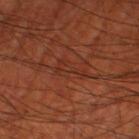Q: Was a biopsy performed?
A: total-body-photography surveillance lesion; no biopsy
Q: What is the anatomic site?
A: the leg
Q: Who is the patient?
A: male, in their 70s
Q: How was this image acquired?
A: 15 mm crop, total-body photography
Q: Illumination type?
A: cross-polarized
Q: How large is the lesion?
A: ~4 mm (longest diameter)